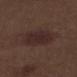Recorded during total-body skin imaging; not selected for excision or biopsy.
Automated image analysis of the tile measured a color-variation rating of about 2.5/10 and peripheral color asymmetry of about 1. The analysis additionally found an automated nevus-likeness rating near 15 out of 100.
The tile uses white-light illumination.
From the right thigh.
A 15 mm close-up extracted from a 3D total-body photography capture.
The lesion's longest dimension is about 4 mm.
The subject is a male aged around 70.